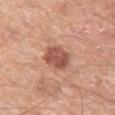notes: no biopsy performed (imaged during a skin exam)
image: total-body-photography crop, ~15 mm field of view
lighting: white-light
body site: the left thigh
lesion size: ~3.5 mm (longest diameter)
image-analysis metrics: a mean CIELAB color near L≈53 a*≈24 b*≈29, a lesion–skin lightness drop of about 13, and a lesion-to-skin contrast of about 8.5 (normalized; higher = more distinct); a border-irregularity rating of about 1.5/10, a within-lesion color-variation index near 3/10, and radial color variation of about 1
patient: male, in their mid- to late 60s